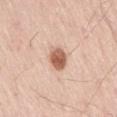workup = no biopsy performed (imaged during a skin exam)
image source = ~15 mm crop, total-body skin-cancer survey
patient = male, about 55 years old
location = the lower back
lesion diameter = ~3 mm (longest diameter)